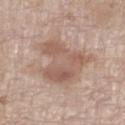<tbp_lesion>
  <biopsy_status>not biopsied; imaged during a skin examination</biopsy_status>
  <lighting>white-light</lighting>
  <patient>
    <sex>male</sex>
    <age_approx>70</age_approx>
  </patient>
  <lesion_size>
    <long_diameter_mm_approx>6.0</long_diameter_mm_approx>
  </lesion_size>
  <site>left lower leg</site>
  <image>
    <source>total-body photography crop</source>
    <field_of_view_mm>15</field_of_view_mm>
  </image>
</tbp_lesion>